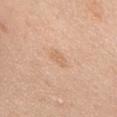Part of a total-body skin-imaging series; this lesion was reviewed on a skin check and was not flagged for biopsy.
A male subject, about 55 years old.
Longest diameter approximately 2.5 mm.
The tile uses white-light illumination.
From the back.
A 15 mm close-up tile from a total-body photography series done for melanoma screening.
Automated tile analysis of the lesion measured a border-irregularity index near 3/10, internal color variation of about 1 on a 0–10 scale, and radial color variation of about 0.5.